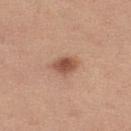Assessment:
The lesion was photographed on a routine skin check and not biopsied; there is no pathology result.
Acquisition and patient details:
The lesion is on the leg. Measured at roughly 3 mm in maximum diameter. Cropped from a total-body skin-imaging series; the visible field is about 15 mm. Automated tile analysis of the lesion measured an average lesion color of about L≈52 a*≈23 b*≈30 (CIELAB) and about 13 CIELAB-L* units darker than the surrounding skin. The software also gave border irregularity of about 2 on a 0–10 scale and peripheral color asymmetry of about 1. It also reported a nevus-likeness score of about 95/100 and a lesion-detection confidence of about 100/100. This is a white-light tile. A female patient in their mid-20s.